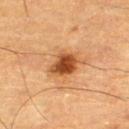<case>
<biopsy_status>not biopsied; imaged during a skin examination</biopsy_status>
<image>
  <source>total-body photography crop</source>
  <field_of_view_mm>15</field_of_view_mm>
</image>
<automated_metrics>
  <vs_skin_darker_L>14.0</vs_skin_darker_L>
</automated_metrics>
<patient>
  <sex>male</sex>
  <age_approx>85+</age_approx>
</patient>
<lesion_size>
  <long_diameter_mm_approx>4.0</long_diameter_mm_approx>
</lesion_size>
<site>right thigh</site>
<lighting>cross-polarized</lighting>
</case>